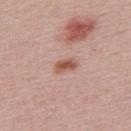Imaged during a routine full-body skin examination; the lesion was not biopsied and no histopathology is available.
The lesion-visualizer software estimated about 12 CIELAB-L* units darker than the surrounding skin and a normalized border contrast of about 9. The software also gave a border-irregularity index near 2/10, a within-lesion color-variation index near 4/10, and radial color variation of about 1.5. And it measured a nevus-likeness score of about 90/100 and a lesion-detection confidence of about 100/100.
Approximately 2.5 mm at its widest.
The patient is a male roughly 30 years of age.
A close-up tile cropped from a whole-body skin photograph, about 15 mm across.
This is a white-light tile.